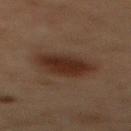The lesion was photographed on a routine skin check and not biopsied; there is no pathology result. A 15 mm close-up extracted from a 3D total-body photography capture. From the mid back. The patient is a male in their 70s. The tile uses cross-polarized illumination. Approximately 6 mm at its widest.